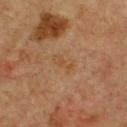Imaged during a routine full-body skin examination; the lesion was not biopsied and no histopathology is available. The recorded lesion diameter is about 3 mm. An algorithmic analysis of the crop reported an area of roughly 3 mm², a shape eccentricity near 0.9, and a shape-asymmetry score of about 0.3 (0 = symmetric). And it measured a border-irregularity rating of about 4/10 and peripheral color asymmetry of about 0. From the front of the torso. A 15 mm close-up extracted from a 3D total-body photography capture. Imaged with cross-polarized lighting. A male patient about 75 years old.